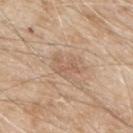workup — catalogued during a skin exam; not biopsied | site — the upper back | image — 15 mm crop, total-body photography | subject — male, aged 78 to 82.